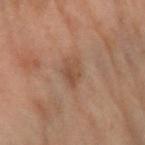Notes:
– notes · imaged on a skin check; not biopsied
– subject · female, roughly 60 years of age
– imaging modality · ~15 mm crop, total-body skin-cancer survey
– body site · the right arm